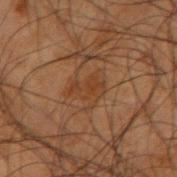Captured during whole-body skin photography for melanoma surveillance; the lesion was not biopsied. The lesion's longest dimension is about 3.5 mm. A 15 mm close-up extracted from a 3D total-body photography capture. A male patient aged around 50. Imaged with cross-polarized lighting. Automated tile analysis of the lesion measured an average lesion color of about L≈30 a*≈17 b*≈27 (CIELAB), a lesion–skin lightness drop of about 5, and a lesion-to-skin contrast of about 5.5 (normalized; higher = more distinct). Located on the arm.